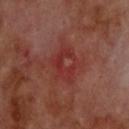Assessment:
Recorded during total-body skin imaging; not selected for excision or biopsy.
Background:
The subject is a male aged around 70. Located on the upper back. Captured under cross-polarized illumination. A roughly 15 mm field-of-view crop from a total-body skin photograph.